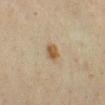Q: Was a biopsy performed?
A: imaged on a skin check; not biopsied